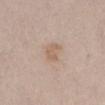{"biopsy_status": "not biopsied; imaged during a skin examination", "site": "abdomen", "image": {"source": "total-body photography crop", "field_of_view_mm": 15}, "patient": {"sex": "female", "age_approx": 65}}